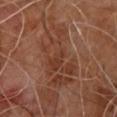Q: Was a biopsy performed?
A: no biopsy performed (imaged during a skin exam)
Q: Lesion size?
A: about 8.5 mm
Q: Patient demographics?
A: male, aged 63–67
Q: How was this image acquired?
A: ~15 mm crop, total-body skin-cancer survey
Q: Illumination type?
A: cross-polarized illumination